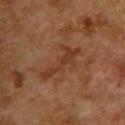notes: no biopsy performed (imaged during a skin exam).